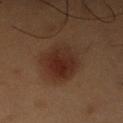* notes: catalogued during a skin exam; not biopsied
* body site: the right upper arm
* acquisition: ~15 mm crop, total-body skin-cancer survey
* subject: male, roughly 55 years of age